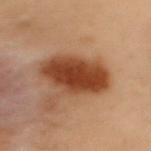| feature | finding |
|---|---|
| notes | imaged on a skin check; not biopsied |
| TBP lesion metrics | a shape eccentricity near 0.85 and a symmetry-axis asymmetry near 0.15 |
| location | the upper back |
| imaging modality | ~15 mm tile from a whole-body skin photo |
| lesion diameter | ≈7.5 mm |
| lighting | cross-polarized |
| patient | female, about 60 years old |